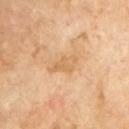| feature | finding |
|---|---|
| acquisition | ~15 mm crop, total-body skin-cancer survey |
| lesion size | about 3.5 mm |
| patient | male, aged 68–72 |
| location | the front of the torso |
| illumination | cross-polarized illumination |
| TBP lesion metrics | about 7 CIELAB-L* units darker than the surrounding skin and a normalized border contrast of about 5.5; a border-irregularity rating of about 4/10, a color-variation rating of about 1.5/10, and a peripheral color-asymmetry measure near 0.5 |